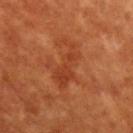Q: Was this lesion biopsied?
A: no biopsy performed (imaged during a skin exam)
Q: How was this image acquired?
A: ~15 mm crop, total-body skin-cancer survey
Q: Patient demographics?
A: female, aged 78 to 82
Q: Where on the body is the lesion?
A: the upper back
Q: Lesion size?
A: ~6 mm (longest diameter)
Q: Automated lesion metrics?
A: a footprint of about 10 mm² and an outline eccentricity of about 0.9 (0 = round, 1 = elongated); a border-irregularity rating of about 6.5/10, internal color variation of about 2 on a 0–10 scale, and peripheral color asymmetry of about 0.5; an automated nevus-likeness rating near 0 out of 100 and a lesion-detection confidence of about 100/100